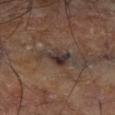<lesion>
  <biopsy_status>not biopsied; imaged during a skin examination</biopsy_status>
  <site>right lower leg</site>
  <lighting>cross-polarized</lighting>
  <lesion_size>
    <long_diameter_mm_approx>4.0</long_diameter_mm_approx>
  </lesion_size>
  <image>
    <source>total-body photography crop</source>
    <field_of_view_mm>15</field_of_view_mm>
  </image>
</lesion>